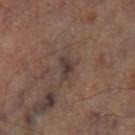Assessment: The lesion was tiled from a total-body skin photograph and was not biopsied. Clinical summary: Cropped from a whole-body photographic skin survey; the tile spans about 15 mm. Approximately 3 mm at its widest. Imaged with cross-polarized lighting. The total-body-photography lesion software estimated an eccentricity of roughly 0.75 and two-axis asymmetry of about 0.3. The analysis additionally found a lesion color around L≈37 a*≈13 b*≈19 in CIELAB, a lesion–skin lightness drop of about 8, and a lesion-to-skin contrast of about 8 (normalized; higher = more distinct). The analysis additionally found an automated nevus-likeness rating near 0 out of 100 and a lesion-detection confidence of about 95/100. From the left lower leg.